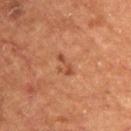Impression:
Imaged during a routine full-body skin examination; the lesion was not biopsied and no histopathology is available.
Image and clinical context:
A male patient aged approximately 55. The tile uses cross-polarized illumination. Located on the upper back. A 15 mm close-up tile from a total-body photography series done for melanoma screening. The lesion-visualizer software estimated a lesion area of about 2.5 mm², a shape eccentricity near 0.9, and a shape-asymmetry score of about 0.5 (0 = symmetric). It also reported a lesion–skin lightness drop of about 8. It also reported a border-irregularity index near 7/10, a within-lesion color-variation index near 0/10, and radial color variation of about 0.